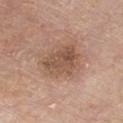Assessment: Captured during whole-body skin photography for melanoma surveillance; the lesion was not biopsied. Clinical summary: A male patient, roughly 80 years of age. The lesion-visualizer software estimated border irregularity of about 2.5 on a 0–10 scale, internal color variation of about 4.5 on a 0–10 scale, and radial color variation of about 1.5. From the chest. A lesion tile, about 15 mm wide, cut from a 3D total-body photograph.